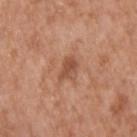Q: Was a biopsy performed?
A: no biopsy performed (imaged during a skin exam)
Q: What lighting was used for the tile?
A: white-light
Q: How was this image acquired?
A: 15 mm crop, total-body photography
Q: What is the anatomic site?
A: the upper back
Q: Who is the patient?
A: male, in their mid- to late 60s